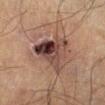notes=catalogued during a skin exam; not biopsied | tile lighting=cross-polarized illumination | location=the leg | patient=male, aged approximately 60 | diameter=≈5 mm | image=~15 mm crop, total-body skin-cancer survey.